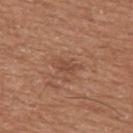biopsy status: imaged on a skin check; not biopsied